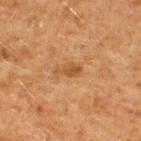notes: catalogued during a skin exam; not biopsied | body site: the upper back | lesion diameter: ~3 mm (longest diameter) | illumination: cross-polarized illumination | image source: ~15 mm crop, total-body skin-cancer survey | patient: male, aged around 50.